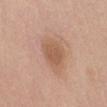Located on the abdomen. About 4 mm across. A female subject aged 58 to 62. A close-up tile cropped from a whole-body skin photograph, about 15 mm across.